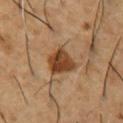The lesion was tiled from a total-body skin photograph and was not biopsied. This is a cross-polarized tile. On the chest. A male patient, aged 53 to 57. An algorithmic analysis of the crop reported an average lesion color of about L≈41 a*≈20 b*≈34 (CIELAB), a lesion–skin lightness drop of about 13, and a lesion-to-skin contrast of about 10.5 (normalized; higher = more distinct). It also reported a classifier nevus-likeness of about 90/100. A 15 mm close-up extracted from a 3D total-body photography capture.